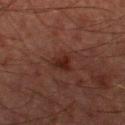Impression: Recorded during total-body skin imaging; not selected for excision or biopsy. Clinical summary: The tile uses cross-polarized illumination. About 3.5 mm across. A lesion tile, about 15 mm wide, cut from a 3D total-body photograph. From the right thigh. The subject is a male in their mid-60s. The lesion-visualizer software estimated an area of roughly 6 mm², an eccentricity of roughly 0.65, and two-axis asymmetry of about 0.35. The software also gave a lesion color around L≈20 a*≈18 b*≈19 in CIELAB, a lesion–skin lightness drop of about 6, and a normalized lesion–skin contrast near 7.